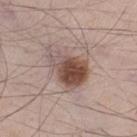Impression: Recorded during total-body skin imaging; not selected for excision or biopsy. Acquisition and patient details: Cropped from a total-body skin-imaging series; the visible field is about 15 mm. Automated tile analysis of the lesion measured a lesion area of about 15 mm² and a symmetry-axis asymmetry near 0.35. And it measured a lesion color around L≈49 a*≈18 b*≈23 in CIELAB and a lesion–skin lightness drop of about 14. The analysis additionally found a detector confidence of about 100 out of 100 that the crop contains a lesion. From the left thigh. A male patient, in their mid- to late 40s. Measured at roughly 5.5 mm in maximum diameter.